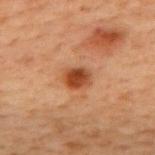Imaged during a routine full-body skin examination; the lesion was not biopsied and no histopathology is available. This image is a 15 mm lesion crop taken from a total-body photograph. A male patient, approximately 70 years of age. The lesion is located on the upper back. The lesion-visualizer software estimated border irregularity of about 1.5 on a 0–10 scale, a color-variation rating of about 3.5/10, and peripheral color asymmetry of about 1. The lesion's longest dimension is about 3 mm. This is a cross-polarized tile.